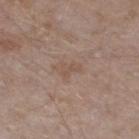Part of a total-body skin-imaging series; this lesion was reviewed on a skin check and was not flagged for biopsy.
Automated tile analysis of the lesion measured a lesion area of about 3.5 mm², an outline eccentricity of about 0.75 (0 = round, 1 = elongated), and a symmetry-axis asymmetry near 0.45. And it measured border irregularity of about 5 on a 0–10 scale, a color-variation rating of about 0/10, and radial color variation of about 0.
A roughly 15 mm field-of-view crop from a total-body skin photograph.
A male subject aged 43–47.
The lesion is on the left lower leg.
Approximately 2.5 mm at its widest.